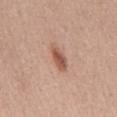Imaged during a routine full-body skin examination; the lesion was not biopsied and no histopathology is available. The lesion is located on the mid back. The tile uses white-light illumination. A female subject, in their mid- to late 60s. About 4 mm across. A roughly 15 mm field-of-view crop from a total-body skin photograph.